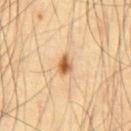{"biopsy_status": "not biopsied; imaged during a skin examination", "site": "abdomen", "image": {"source": "total-body photography crop", "field_of_view_mm": 15}, "lighting": "cross-polarized", "lesion_size": {"long_diameter_mm_approx": 2.5}, "patient": {"sex": "male", "age_approx": 55}}